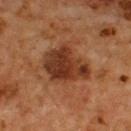biopsy status=no biopsy performed (imaged during a skin exam)
location=the upper back
patient=male, aged 63–67
imaging modality=~15 mm crop, total-body skin-cancer survey
lesion size=≈6 mm
tile lighting=cross-polarized